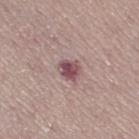workup: no biopsy performed (imaged during a skin exam)
subject: female, in their mid- to late 50s
body site: the right thigh
automated lesion analysis: a lesion color around L≈49 a*≈23 b*≈15 in CIELAB, about 13 CIELAB-L* units darker than the surrounding skin, and a normalized lesion–skin contrast near 10; a border-irregularity index near 2/10, a color-variation rating of about 4/10, and a peripheral color-asymmetry measure near 1.5; lesion-presence confidence of about 100/100
size: ~2.5 mm (longest diameter)
illumination: white-light
image source: ~15 mm tile from a whole-body skin photo